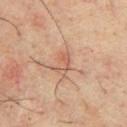{
  "biopsy_status": "not biopsied; imaged during a skin examination",
  "automated_metrics": {
    "area_mm2_approx": 4.5,
    "cielab_L": 54,
    "cielab_a": 20,
    "cielab_b": 28,
    "vs_skin_darker_L": 7.0,
    "vs_skin_contrast_norm": 5.0
  },
  "patient": {
    "sex": "male",
    "age_approx": 35
  },
  "site": "chest",
  "lesion_size": {
    "long_diameter_mm_approx": 3.0
  },
  "image": {
    "source": "total-body photography crop",
    "field_of_view_mm": 15
  }
}